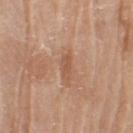| key | value |
|---|---|
| follow-up | imaged on a skin check; not biopsied |
| image | ~15 mm crop, total-body skin-cancer survey |
| location | the arm |
| subject | male, approximately 80 years of age |